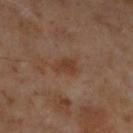Clinical impression:
Imaged during a routine full-body skin examination; the lesion was not biopsied and no histopathology is available.
Background:
On the left lower leg. A male subject about 60 years old. Imaged with cross-polarized lighting. A 15 mm crop from a total-body photograph taken for skin-cancer surveillance. Measured at roughly 2.5 mm in maximum diameter.